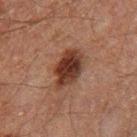This lesion was catalogued during total-body skin photography and was not selected for biopsy.
Cropped from a whole-body photographic skin survey; the tile spans about 15 mm.
A male patient, about 60 years old.
Located on the right thigh.
This is a cross-polarized tile.